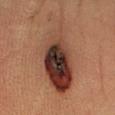Part of a total-body skin-imaging series; this lesion was reviewed on a skin check and was not flagged for biopsy.
Approximately 10 mm at its widest.
The lesion is located on the left forearm.
Captured under cross-polarized illumination.
This image is a 15 mm lesion crop taken from a total-body photograph.
A male patient aged 63 to 67.